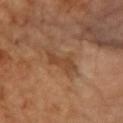follow-up: imaged on a skin check; not biopsied
image: ~15 mm tile from a whole-body skin photo
site: the right forearm
size: about 3.5 mm
lighting: cross-polarized illumination
patient: female, aged around 70